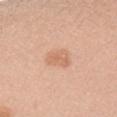workup = no biopsy performed (imaged during a skin exam)
patient = female, aged around 25
acquisition = ~15 mm crop, total-body skin-cancer survey
illumination = white-light illumination
site = the left forearm